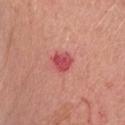| key | value |
|---|---|
| workup | catalogued during a skin exam; not biopsied |
| patient | female, aged approximately 60 |
| site | the head or neck |
| imaging modality | 15 mm crop, total-body photography |
| automated lesion analysis | an average lesion color of about L≈52 a*≈38 b*≈26 (CIELAB), about 11 CIELAB-L* units darker than the surrounding skin, and a normalized lesion–skin contrast near 8; a color-variation rating of about 2.5/10 and radial color variation of about 1; a lesion-detection confidence of about 100/100 |
| lesion size | ~3 mm (longest diameter) |